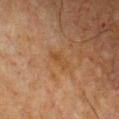Assessment:
No biopsy was performed on this lesion — it was imaged during a full skin examination and was not determined to be concerning.
Context:
A close-up tile cropped from a whole-body skin photograph, about 15 mm across. The lesion is located on the chest. An algorithmic analysis of the crop reported a lesion area of about 3.5 mm², a shape eccentricity near 0.9, and two-axis asymmetry of about 0.3. And it measured border irregularity of about 3.5 on a 0–10 scale, a within-lesion color-variation index near 1/10, and a peripheral color-asymmetry measure near 0. The analysis additionally found a classifier nevus-likeness of about 0/100 and a detector confidence of about 100 out of 100 that the crop contains a lesion. Measured at roughly 3 mm in maximum diameter. The tile uses cross-polarized illumination. A male subject, aged around 65.